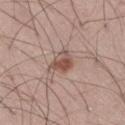  biopsy_status: not biopsied; imaged during a skin examination
  patient:
    sex: male
    age_approx: 55
  lighting: white-light
  lesion_size:
    long_diameter_mm_approx: 3.0
  image:
    source: total-body photography crop
    field_of_view_mm: 15
  automated_metrics:
    cielab_L: 51
    cielab_a: 19
    cielab_b: 25
    vs_skin_contrast_norm: 8.0
    border_irregularity_0_10: 2.5
    peripheral_color_asymmetry: 2.0
    nevus_likeness_0_100: 90